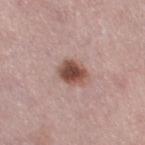  biopsy_status: not biopsied; imaged during a skin examination
  automated_metrics:
    area_mm2_approx: 7.0
    eccentricity: 0.45
    shape_asymmetry: 0.15
    border_irregularity_0_10: 1.5
    color_variation_0_10: 5.0
    peripheral_color_asymmetry: 1.5
    nevus_likeness_0_100: 100
    lesion_detection_confidence_0_100: 100
  site: right thigh
  patient:
    sex: female
    age_approx: 50
  image:
    source: total-body photography crop
    field_of_view_mm: 15